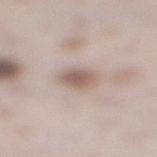Cropped from a whole-body photographic skin survey; the tile spans about 15 mm. A male subject roughly 40 years of age. Automated image analysis of the tile measured a border-irregularity index near 1/10 and radial color variation of about 0.5. And it measured an automated nevus-likeness rating near 90 out of 100 and a detector confidence of about 100 out of 100 that the crop contains a lesion. On the right lower leg.